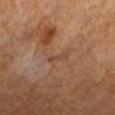Assessment: This lesion was catalogued during total-body skin photography and was not selected for biopsy. Context: Automated image analysis of the tile measured a footprint of about 1.5 mm², a shape eccentricity near 0.95, and a shape-asymmetry score of about 0.4 (0 = symmetric). It also reported a mean CIELAB color near L≈42 a*≈19 b*≈28 and a normalized border contrast of about 5. A female patient, about 65 years old. Measured at roughly 2.5 mm in maximum diameter. The lesion is on the left lower leg. A close-up tile cropped from a whole-body skin photograph, about 15 mm across.